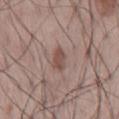The lesion is on the mid back.
Cropped from a total-body skin-imaging series; the visible field is about 15 mm.
The lesion's longest dimension is about 3 mm.
A male patient aged around 45.
Captured under white-light illumination.